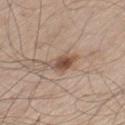Assessment:
Part of a total-body skin-imaging series; this lesion was reviewed on a skin check and was not flagged for biopsy.
Clinical summary:
Imaged with white-light lighting. Automated tile analysis of the lesion measured an area of roughly 5.5 mm². The analysis additionally found border irregularity of about 2.5 on a 0–10 scale, internal color variation of about 5 on a 0–10 scale, and radial color variation of about 1.5. From the left thigh. A close-up tile cropped from a whole-body skin photograph, about 15 mm across. A male subject, aged approximately 60. About 3.5 mm across.